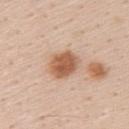Recorded during total-body skin imaging; not selected for excision or biopsy. A male patient, about 60 years old. A region of skin cropped from a whole-body photographic capture, roughly 15 mm wide. On the upper back.